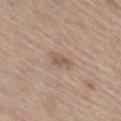workup: imaged on a skin check; not biopsied | patient: male, aged 68 to 72 | illumination: white-light illumination | imaging modality: total-body-photography crop, ~15 mm field of view | automated metrics: a shape eccentricity near 0.85 and two-axis asymmetry of about 0.35 | location: the right lower leg | size: about 3 mm.